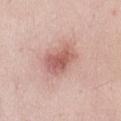Notes:
• imaging modality · ~15 mm tile from a whole-body skin photo
• patient · female, in their 40s
• lesion diameter · ≈4.5 mm
• site · the front of the torso